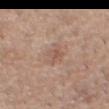This lesion was catalogued during total-body skin photography and was not selected for biopsy. Measured at roughly 3 mm in maximum diameter. Automated image analysis of the tile measured border irregularity of about 3.5 on a 0–10 scale, a within-lesion color-variation index near 3.5/10, and peripheral color asymmetry of about 1. And it measured a nevus-likeness score of about 0/100 and a detector confidence of about 100 out of 100 that the crop contains a lesion. The lesion is located on the head or neck. A roughly 15 mm field-of-view crop from a total-body skin photograph. The subject is a male aged around 35. The tile uses white-light illumination.